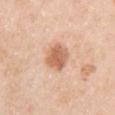Impression: Part of a total-body skin-imaging series; this lesion was reviewed on a skin check and was not flagged for biopsy. Background: A female patient roughly 50 years of age. The lesion is on the right upper arm. A close-up tile cropped from a whole-body skin photograph, about 15 mm across. The tile uses white-light illumination. The total-body-photography lesion software estimated an eccentricity of roughly 0.4 and a symmetry-axis asymmetry near 0.2. The software also gave a nevus-likeness score of about 95/100 and lesion-presence confidence of about 100/100. About 3.5 mm across.